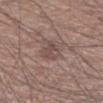Findings:
* patient: male, aged 53–57
* lesion size: ~2.5 mm (longest diameter)
* illumination: white-light illumination
* image source: 15 mm crop, total-body photography
* body site: the right forearm